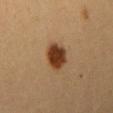Recorded during total-body skin imaging; not selected for excision or biopsy. Located on the right upper arm. A 15 mm close-up extracted from a 3D total-body photography capture. Automated tile analysis of the lesion measured a lesion color around L≈35 a*≈19 b*≈31 in CIELAB, roughly 15 lightness units darker than nearby skin, and a normalized lesion–skin contrast near 13. The software also gave a border-irregularity index near 1.5/10, a within-lesion color-variation index near 4/10, and peripheral color asymmetry of about 1. The subject is a female aged 28–32. Imaged with cross-polarized lighting. Approximately 3.5 mm at its widest.